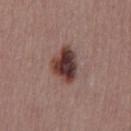• biopsy status — catalogued during a skin exam; not biopsied
• imaging modality — ~15 mm crop, total-body skin-cancer survey
• site — the front of the torso
• diameter — ≈4.5 mm
• subject — male, roughly 40 years of age
• tile lighting — white-light